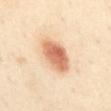  biopsy_status: not biopsied; imaged during a skin examination
  image:
    source: total-body photography crop
    field_of_view_mm: 15
  lesion_size:
    long_diameter_mm_approx: 4.5
  patient:
    sex: female
    age_approx: 55
  site: abdomen
  automated_metrics:
    color_variation_0_10: 5.5
    peripheral_color_asymmetry: 1.5
  lighting: cross-polarized